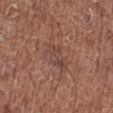follow-up — catalogued during a skin exam; not biopsied
imaging modality — ~15 mm crop, total-body skin-cancer survey
lesion diameter — ~4 mm (longest diameter)
patient — female, in their 50s
automated metrics — an area of roughly 6.5 mm² and a symmetry-axis asymmetry near 0.4; a nevus-likeness score of about 0/100 and a lesion-detection confidence of about 95/100
body site — the leg